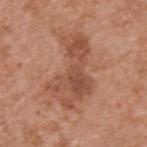Impression:
This lesion was catalogued during total-body skin photography and was not selected for biopsy.
Clinical summary:
Cropped from a whole-body photographic skin survey; the tile spans about 15 mm. The subject is a male in their mid- to late 60s. From the upper back. About 8 mm across.